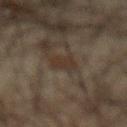Located on the abdomen. Automated image analysis of the tile measured a shape eccentricity near 0.65 and a shape-asymmetry score of about 0.3 (0 = symmetric). It also reported a mean CIELAB color near L≈26 a*≈11 b*≈19 and a lesion–skin lightness drop of about 5. And it measured a border-irregularity rating of about 3/10 and a color-variation rating of about 2/10. Imaged with cross-polarized lighting. The patient is a male aged approximately 65. A 15 mm crop from a total-body photograph taken for skin-cancer surveillance.